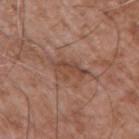Case summary:
– workup · no biopsy performed (imaged during a skin exam)
– lighting · white-light illumination
– lesion size · about 4 mm
– patient · male, in their mid- to late 70s
– automated metrics · a mean CIELAB color near L≈46 a*≈21 b*≈28, about 8 CIELAB-L* units darker than the surrounding skin, and a normalized lesion–skin contrast near 6.5; a classifier nevus-likeness of about 0/100 and lesion-presence confidence of about 85/100
– anatomic site · the right upper arm
– imaging modality · ~15 mm tile from a whole-body skin photo